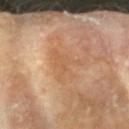Assessment:
No biopsy was performed on this lesion — it was imaged during a full skin examination and was not determined to be concerning.
Acquisition and patient details:
The lesion's longest dimension is about 4.5 mm. The lesion-visualizer software estimated a border-irregularity index near 9.5/10, a within-lesion color-variation index near 1/10, and peripheral color asymmetry of about 0.5. The software also gave a nevus-likeness score of about 0/100. A lesion tile, about 15 mm wide, cut from a 3D total-body photograph. A female subject, approximately 75 years of age. The tile uses cross-polarized illumination. Located on the right forearm.